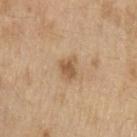This lesion was catalogued during total-body skin photography and was not selected for biopsy. A male patient, approximately 70 years of age. Located on the left upper arm. A close-up tile cropped from a whole-body skin photograph, about 15 mm across.